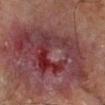{"biopsy_status": "not biopsied; imaged during a skin examination", "automated_metrics": {"area_mm2_approx": 165.0, "eccentricity": 0.55, "shape_asymmetry": 0.4, "cielab_L": 32, "cielab_a": 18, "cielab_b": 17, "vs_skin_darker_L": 8.0, "vs_skin_contrast_norm": 8.5}, "patient": {"sex": "male", "age_approx": 65}, "lesion_size": {"long_diameter_mm_approx": 18.5}, "site": "left lower leg", "image": {"source": "total-body photography crop", "field_of_view_mm": 15}}